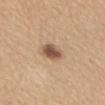Captured during whole-body skin photography for melanoma surveillance; the lesion was not biopsied. The recorded lesion diameter is about 3 mm. Cropped from a whole-body photographic skin survey; the tile spans about 15 mm. The lesion is located on the mid back. A female subject, in their 60s.